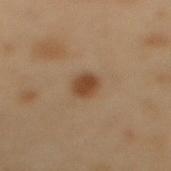follow-up=no biopsy performed (imaged during a skin exam)
imaging modality=~15 mm crop, total-body skin-cancer survey
subject=male, approximately 55 years of age
body site=the mid back